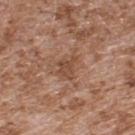follow-up: imaged on a skin check; not biopsied | lesion diameter: ≈3 mm | tile lighting: white-light | imaging modality: ~15 mm tile from a whole-body skin photo | site: the back | patient: male, aged approximately 55.